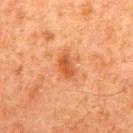follow-up: no biopsy performed (imaged during a skin exam) | image: 15 mm crop, total-body photography | body site: the mid back | subject: male, in their 80s | tile lighting: cross-polarized | diameter: about 3 mm | automated lesion analysis: border irregularity of about 2 on a 0–10 scale, a within-lesion color-variation index near 2.5/10, and radial color variation of about 1; lesion-presence confidence of about 100/100.